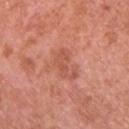Assessment: No biopsy was performed on this lesion — it was imaged during a full skin examination and was not determined to be concerning. Context: The subject is a male approximately 70 years of age. A lesion tile, about 15 mm wide, cut from a 3D total-body photograph. Imaged with white-light lighting. The lesion is located on the chest. About 3.5 mm across. The total-body-photography lesion software estimated a lesion area of about 5 mm² and an outline eccentricity of about 0.85 (0 = round, 1 = elongated). It also reported a mean CIELAB color near L≈55 a*≈29 b*≈32, a lesion–skin lightness drop of about 8, and a lesion-to-skin contrast of about 5.5 (normalized; higher = more distinct). The software also gave a border-irregularity index near 7.5/10, internal color variation of about 1.5 on a 0–10 scale, and peripheral color asymmetry of about 0.5.